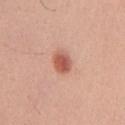| feature | finding |
|---|---|
| site | the front of the torso |
| subject | male, aged 28 to 32 |
| lighting | white-light illumination |
| lesion diameter | ~3 mm (longest diameter) |
| imaging modality | ~15 mm crop, total-body skin-cancer survey |